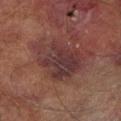workup: total-body-photography surveillance lesion; no biopsy | image source: ~15 mm crop, total-body skin-cancer survey | size: about 6.5 mm | image-analysis metrics: a border-irregularity index near 4/10, a within-lesion color-variation index near 4/10, and peripheral color asymmetry of about 1.5; a nevus-likeness score of about 0/100 and lesion-presence confidence of about 100/100 | subject: male, in their mid-70s | illumination: cross-polarized | site: the left lower leg.